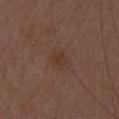Clinical impression:
Recorded during total-body skin imaging; not selected for excision or biopsy.
Context:
A region of skin cropped from a whole-body photographic capture, roughly 15 mm wide. From the head or neck. This is a white-light tile. Automated image analysis of the tile measured a shape eccentricity near 0.7 and a shape-asymmetry score of about 0.2 (0 = symmetric). The software also gave an automated nevus-likeness rating near 5 out of 100. The recorded lesion diameter is about 2.5 mm. The subject is a female aged 28–32.